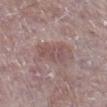- notes — catalogued during a skin exam; not biopsied
- lesion diameter — ~4 mm (longest diameter)
- anatomic site — the right lower leg
- image — total-body-photography crop, ~15 mm field of view
- illumination — white-light
- automated lesion analysis — border irregularity of about 4.5 on a 0–10 scale, a within-lesion color-variation index near 2.5/10, and radial color variation of about 1
- subject — male, in their mid- to late 70s